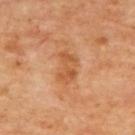  biopsy_status: not biopsied; imaged during a skin examination
  image:
    source: total-body photography crop
    field_of_view_mm: 15
  site: upper back
  patient:
    age_approx: 65
  lesion_size:
    long_diameter_mm_approx: 4.0